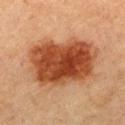biopsy status — no biopsy performed (imaged during a skin exam)
patient — female, approximately 60 years of age
image — ~15 mm tile from a whole-body skin photo
lesion diameter — ~8.5 mm (longest diameter)
illumination — cross-polarized illumination
image-analysis metrics — a lesion area of about 42 mm², an outline eccentricity of about 0.7 (0 = round, 1 = elongated), and a shape-asymmetry score of about 0.15 (0 = symmetric); radial color variation of about 2.5; an automated nevus-likeness rating near 100 out of 100 and a lesion-detection confidence of about 100/100
site — the chest